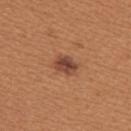follow-up: imaged on a skin check; not biopsied
site: the back
image source: ~15 mm tile from a whole-body skin photo
illumination: white-light
subject: female, aged 38 to 42
lesion diameter: ~3 mm (longest diameter)